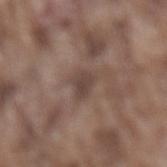Clinical summary:
On the lower back. The subject is a male about 75 years old. The recorded lesion diameter is about 2.5 mm. The tile uses white-light illumination. A region of skin cropped from a whole-body photographic capture, roughly 15 mm wide.